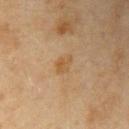| key | value |
|---|---|
| biopsy status | total-body-photography surveillance lesion; no biopsy |
| patient | female, aged 58–62 |
| image | ~15 mm crop, total-body skin-cancer survey |
| lesion size | ~2.5 mm (longest diameter) |
| location | the right upper arm |